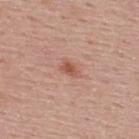<case>
<biopsy_status>not biopsied; imaged during a skin examination</biopsy_status>
<patient>
  <sex>male</sex>
  <age_approx>55</age_approx>
</patient>
<site>upper back</site>
<image>
  <source>total-body photography crop</source>
  <field_of_view_mm>15</field_of_view_mm>
</image>
</case>